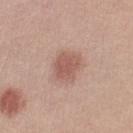Recorded during total-body skin imaging; not selected for excision or biopsy.
The recorded lesion diameter is about 3.5 mm.
The subject is a female aged around 20.
A 15 mm crop from a total-body photograph taken for skin-cancer surveillance.
Located on the left lower leg.